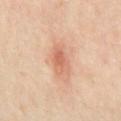location: the chest | lesion diameter: ~4 mm (longest diameter) | image source: 15 mm crop, total-body photography | subject: male, about 65 years old | lighting: cross-polarized.